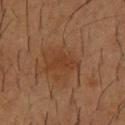A male subject aged 53–57. The lesion is on the chest. A 15 mm close-up tile from a total-body photography series done for melanoma screening.